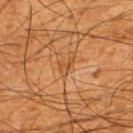• follow-up · total-body-photography surveillance lesion; no biopsy
• body site · the upper back
• imaging modality · ~15 mm crop, total-body skin-cancer survey
• lesion diameter · about 2.5 mm
• tile lighting · cross-polarized
• patient · male, aged approximately 65